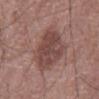Findings:
- image source: ~15 mm tile from a whole-body skin photo
- lesion size: ≈5.5 mm
- illumination: white-light illumination
- site: the chest
- subject: male, about 60 years old